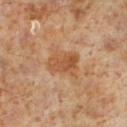notes — imaged on a skin check; not biopsied
image-analysis metrics — a lesion area of about 8.5 mm², a shape eccentricity near 0.75, and a shape-asymmetry score of about 0.2 (0 = symmetric); a lesion-detection confidence of about 100/100
image source — ~15 mm tile from a whole-body skin photo
lesion diameter — ≈4 mm
patient — male, about 60 years old
location — the left lower leg